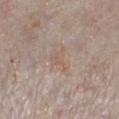Clinical impression:
Captured during whole-body skin photography for melanoma surveillance; the lesion was not biopsied.